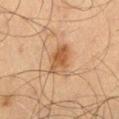Assessment:
Captured during whole-body skin photography for melanoma surveillance; the lesion was not biopsied.
Context:
A close-up tile cropped from a whole-body skin photograph, about 15 mm across. From the right thigh. The subject is a male in their 70s. Measured at roughly 3.5 mm in maximum diameter. An algorithmic analysis of the crop reported a footprint of about 8.5 mm², an eccentricity of roughly 0.65, and a symmetry-axis asymmetry near 0.25. The software also gave a border-irregularity index near 2/10, internal color variation of about 4.5 on a 0–10 scale, and a peripheral color-asymmetry measure near 1.5.